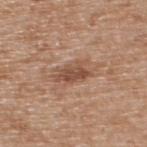About 4 mm across. This is a white-light tile. The patient is a male in their mid- to late 60s. Cropped from a whole-body photographic skin survey; the tile spans about 15 mm. The lesion is on the upper back.